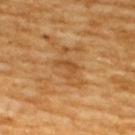No biopsy was performed on this lesion — it was imaged during a full skin examination and was not determined to be concerning.
A male patient, aged approximately 60.
This is a cross-polarized tile.
Approximately 2.5 mm at its widest.
A 15 mm crop from a total-body photograph taken for skin-cancer surveillance.
The total-body-photography lesion software estimated a mean CIELAB color near L≈48 a*≈22 b*≈40, about 7 CIELAB-L* units darker than the surrounding skin, and a normalized lesion–skin contrast near 5.5.
Located on the upper back.